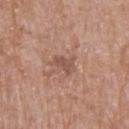follow-up: total-body-photography surveillance lesion; no biopsy
size: ≈3 mm
illumination: white-light illumination
patient: male, in their mid-50s
automated lesion analysis: a lesion-detection confidence of about 100/100
acquisition: ~15 mm crop, total-body skin-cancer survey